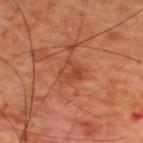Recorded during total-body skin imaging; not selected for excision or biopsy. Cropped from a whole-body photographic skin survey; the tile spans about 15 mm. A male subject, approximately 60 years of age. This is a cross-polarized tile. The lesion is located on the upper back. The recorded lesion diameter is about 3 mm. The total-body-photography lesion software estimated internal color variation of about 4 on a 0–10 scale and peripheral color asymmetry of about 1.5.